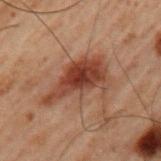No biopsy was performed on this lesion — it was imaged during a full skin examination and was not determined to be concerning.
Longest diameter approximately 7 mm.
A region of skin cropped from a whole-body photographic capture, roughly 15 mm wide.
The patient is a male about 60 years old.
The lesion is on the left upper arm.